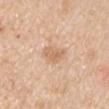This lesion was catalogued during total-body skin photography and was not selected for biopsy. The subject is a male aged 58 to 62. The lesion-visualizer software estimated an area of roughly 5.5 mm². And it measured a classifier nevus-likeness of about 15/100. The lesion is on the right upper arm. This is a white-light tile. A 15 mm crop from a total-body photograph taken for skin-cancer surveillance. Measured at roughly 3 mm in maximum diameter.